The lesion is on the front of the torso. The subject is a female aged around 65. A 15 mm crop from a total-body photograph taken for skin-cancer surveillance:
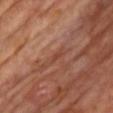The lesion was biopsied, and histopathology showed an actinic keratosis (borderline).